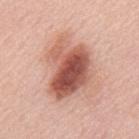workup — catalogued during a skin exam; not biopsied
automated lesion analysis — two-axis asymmetry of about 0.4; a classifier nevus-likeness of about 85/100 and a lesion-detection confidence of about 100/100
subject — male, roughly 55 years of age
acquisition — 15 mm crop, total-body photography
site — the mid back
lesion diameter — about 7 mm